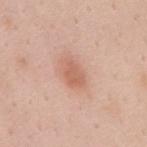biopsy_status: not biopsied; imaged during a skin examination
site: mid back
patient:
  sex: female
  age_approx: 50
image:
  source: total-body photography crop
  field_of_view_mm: 15
lighting: white-light
automated_metrics:
  eccentricity: 0.8
  shape_asymmetry: 0.2
  border_irregularity_0_10: 2.0
  color_variation_0_10: 2.0
  lesion_detection_confidence_0_100: 100